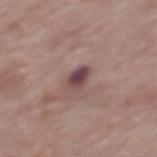Notes:
– workup — catalogued during a skin exam; not biopsied
– diameter — ≈2.5 mm
– patient — female, aged around 65
– site — the upper back
– imaging modality — total-body-photography crop, ~15 mm field of view
– tile lighting — white-light